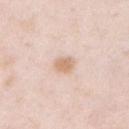No biopsy was performed on this lesion — it was imaged during a full skin examination and was not determined to be concerning. This is a white-light tile. A male subject aged around 35. About 2.5 mm across. The lesion is on the chest. A roughly 15 mm field-of-view crop from a total-body skin photograph.